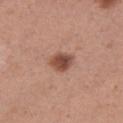<tbp_lesion>
<biopsy_status>not biopsied; imaged during a skin examination</biopsy_status>
<automated_metrics>
  <area_mm2_approx>6.0</area_mm2_approx>
  <eccentricity>0.6</eccentricity>
  <shape_asymmetry>0.2</shape_asymmetry>
  <cielab_L>49</cielab_L>
  <cielab_a>22</cielab_a>
  <cielab_b>28</cielab_b>
  <vs_skin_darker_L>13.0</vs_skin_darker_L>
  <vs_skin_contrast_norm>9.5</vs_skin_contrast_norm>
</automated_metrics>
<patient>
  <sex>female</sex>
  <age_approx>30</age_approx>
</patient>
<lesion_size>
  <long_diameter_mm_approx>3.0</long_diameter_mm_approx>
</lesion_size>
<site>right thigh</site>
<lighting>white-light</lighting>
<image>
  <source>total-body photography crop</source>
  <field_of_view_mm>15</field_of_view_mm>
</image>
</tbp_lesion>